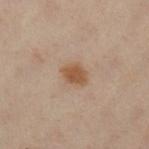Recorded during total-body skin imaging; not selected for excision or biopsy.
Captured under cross-polarized illumination.
Automated tile analysis of the lesion measured a lesion area of about 5.5 mm², an outline eccentricity of about 0.7 (0 = round, 1 = elongated), and a shape-asymmetry score of about 0.2 (0 = symmetric).
The lesion is on the right leg.
A female subject about 60 years old.
A 15 mm close-up extracted from a 3D total-body photography capture.